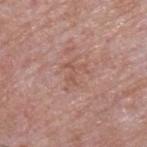<record>
  <image>
    <source>total-body photography crop</source>
    <field_of_view_mm>15</field_of_view_mm>
  </image>
  <site>upper back</site>
  <automated_metrics>
    <area_mm2_approx>5.0</area_mm2_approx>
    <eccentricity>0.65</eccentricity>
    <shape_asymmetry>0.55</shape_asymmetry>
    <border_irregularity_0_10>9.0</border_irregularity_0_10>
    <color_variation_0_10>0.0</color_variation_0_10>
    <peripheral_color_asymmetry>0.0</peripheral_color_asymmetry>
    <nevus_likeness_0_100>0</nevus_likeness_0_100>
  </automated_metrics>
  <patient>
    <sex>male</sex>
    <age_approx>65</age_approx>
  </patient>
</record>